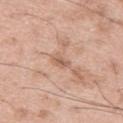A male subject, aged approximately 50. The lesion is on the left thigh. Approximately 2.5 mm at its widest. This is a white-light tile. A roughly 15 mm field-of-view crop from a total-body skin photograph.